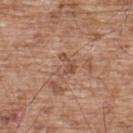Notes:
– notes — catalogued during a skin exam; not biopsied
– anatomic site — the upper back
– lesion size — about 2.5 mm
– lighting — white-light illumination
– TBP lesion metrics — a mean CIELAB color near L≈51 a*≈21 b*≈28 and about 8 CIELAB-L* units darker than the surrounding skin; a border-irregularity rating of about 5.5/10 and a peripheral color-asymmetry measure near 1.5; an automated nevus-likeness rating near 0 out of 100 and a lesion-detection confidence of about 95/100
– subject — male, in their mid-50s
– image — total-body-photography crop, ~15 mm field of view